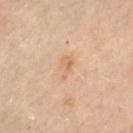A female patient approximately 60 years of age.
The lesion is on the left thigh.
A roughly 15 mm field-of-view crop from a total-body skin photograph.